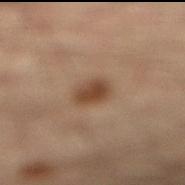Part of a total-body skin-imaging series; this lesion was reviewed on a skin check and was not flagged for biopsy.